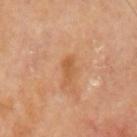{"biopsy_status": "not biopsied; imaged during a skin examination", "lighting": "cross-polarized", "automated_metrics": {"cielab_L": 54, "cielab_a": 23, "cielab_b": 38, "vs_skin_darker_L": 8.0, "vs_skin_contrast_norm": 6.0, "border_irregularity_0_10": 2.5, "color_variation_0_10": 1.0, "peripheral_color_asymmetry": 0.0}, "image": {"source": "total-body photography crop", "field_of_view_mm": 15}, "patient": {"sex": "male", "age_approx": 70}, "site": "left upper arm"}